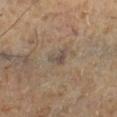– follow-up — catalogued during a skin exam; not biopsied
– imaging modality — ~15 mm tile from a whole-body skin photo
– site — the right lower leg
– subject — male, about 55 years old
– illumination — cross-polarized illumination